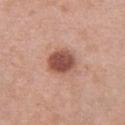follow-up: no biopsy performed (imaged during a skin exam)
subject: female, roughly 40 years of age
TBP lesion metrics: an outline eccentricity of about 0.5 (0 = round, 1 = elongated) and a shape-asymmetry score of about 0.1 (0 = symmetric); a border-irregularity rating of about 1/10 and internal color variation of about 3.5 on a 0–10 scale
location: the chest
acquisition: 15 mm crop, total-body photography
illumination: white-light illumination
size: ~3.5 mm (longest diameter)